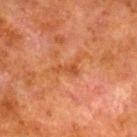| field | value |
|---|---|
| workup | total-body-photography surveillance lesion; no biopsy |
| location | the leg |
| diameter | about 3.5 mm |
| illumination | cross-polarized illumination |
| patient | male, in their 80s |
| image source | ~15 mm crop, total-body skin-cancer survey |